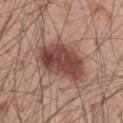Part of a total-body skin-imaging series; this lesion was reviewed on a skin check and was not flagged for biopsy. Located on the back. A male subject about 60 years old. A 15 mm close-up extracted from a 3D total-body photography capture. The tile uses white-light illumination. The total-body-photography lesion software estimated a shape-asymmetry score of about 0.25 (0 = symmetric). It also reported a lesion color around L≈46 a*≈22 b*≈24 in CIELAB, roughly 14 lightness units darker than nearby skin, and a normalized lesion–skin contrast near 10. It also reported lesion-presence confidence of about 100/100. Longest diameter approximately 7 mm.